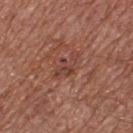<lesion>
<biopsy_status>not biopsied; imaged during a skin examination</biopsy_status>
<lesion_size>
  <long_diameter_mm_approx>4.0</long_diameter_mm_approx>
</lesion_size>
<patient>
  <sex>male</sex>
  <age_approx>65</age_approx>
</patient>
<site>back</site>
<image>
  <source>total-body photography crop</source>
  <field_of_view_mm>15</field_of_view_mm>
</image>
</lesion>